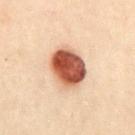Notes:
- notes — catalogued during a skin exam; not biopsied
- diameter — ≈4.5 mm
- patient — female, roughly 30 years of age
- image source — 15 mm crop, total-body photography
- body site — the abdomen